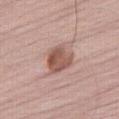Imaged during a routine full-body skin examination; the lesion was not biopsied and no histopathology is available. A 15 mm close-up tile from a total-body photography series done for melanoma screening. This is a white-light tile. A male patient aged 63 to 67. From the abdomen. The recorded lesion diameter is about 3.5 mm.